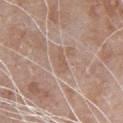Cropped from a whole-body photographic skin survey; the tile spans about 15 mm.
The lesion is on the chest.
The patient is a male aged 78 to 82.